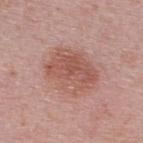Assessment:
Part of a total-body skin-imaging series; this lesion was reviewed on a skin check and was not flagged for biopsy.
Context:
Longest diameter approximately 5.5 mm. Imaged with white-light lighting. A close-up tile cropped from a whole-body skin photograph, about 15 mm across. On the back. A male patient approximately 40 years of age.